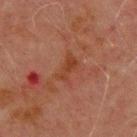Approximately 3.5 mm at its widest.
From the chest.
A male patient, aged 68 to 72.
This is a cross-polarized tile.
A lesion tile, about 15 mm wide, cut from a 3D total-body photograph.
The lesion-visualizer software estimated an average lesion color of about L≈34 a*≈23 b*≈28 (CIELAB), about 6 CIELAB-L* units darker than the surrounding skin, and a normalized border contrast of about 6.5.